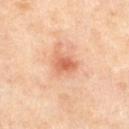Q: Was this lesion biopsied?
A: catalogued during a skin exam; not biopsied
Q: What is the imaging modality?
A: ~15 mm tile from a whole-body skin photo
Q: Lesion size?
A: about 3 mm
Q: What is the anatomic site?
A: the left thigh
Q: Illumination type?
A: cross-polarized illumination
Q: Patient demographics?
A: female, aged 48–52